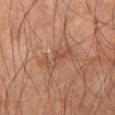- biopsy status: imaged on a skin check; not biopsied
- anatomic site: the leg
- patient: male, aged approximately 55
- image: 15 mm crop, total-body photography